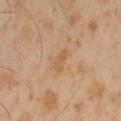follow-up: imaged on a skin check; not biopsied
subject: male, aged approximately 60
image: ~15 mm tile from a whole-body skin photo
tile lighting: cross-polarized
automated lesion analysis: border irregularity of about 3 on a 0–10 scale, a color-variation rating of about 0.5/10, and radial color variation of about 0
body site: the left thigh